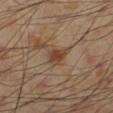{
  "biopsy_status": "not biopsied; imaged during a skin examination",
  "site": "left lower leg",
  "lesion_size": {
    "long_diameter_mm_approx": 2.5
  },
  "patient": {
    "sex": "male",
    "age_approx": 55
  },
  "lighting": "cross-polarized",
  "image": {
    "source": "total-body photography crop",
    "field_of_view_mm": 15
  },
  "automated_metrics": {
    "area_mm2_approx": 4.5,
    "eccentricity": 0.55,
    "shape_asymmetry": 0.3,
    "cielab_L": 42,
    "cielab_a": 17,
    "cielab_b": 29,
    "vs_skin_darker_L": 10.0,
    "vs_skin_contrast_norm": 8.0,
    "border_irregularity_0_10": 2.5,
    "peripheral_color_asymmetry": 0.5
  }
}